The patient is a male roughly 55 years of age. On the arm. A lesion tile, about 15 mm wide, cut from a 3D total-body photograph: 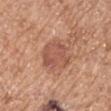The tile uses white-light illumination. The recorded lesion diameter is about 4.5 mm. Histopathological examination showed a dysplastic (Clark) nevus — a benign skin lesion.